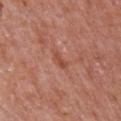workup: catalogued during a skin exam; not biopsied
automated metrics: a footprint of about 3 mm², an eccentricity of roughly 0.9, and a shape-asymmetry score of about 0.3 (0 = symmetric); a border-irregularity index near 3.5/10, a within-lesion color-variation index near 0/10, and radial color variation of about 0
patient: male, in their mid-60s
tile lighting: white-light illumination
location: the chest
acquisition: ~15 mm crop, total-body skin-cancer survey
diameter: ≈2.5 mm